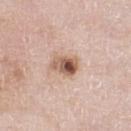Notes:
• follow-up: total-body-photography surveillance lesion; no biopsy
• image: ~15 mm tile from a whole-body skin photo
• subject: male, aged 78–82
• site: the left lower leg